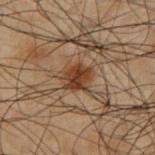Q: Was this lesion biopsied?
A: imaged on a skin check; not biopsied
Q: What are the patient's age and sex?
A: male, aged 48 to 52
Q: What is the imaging modality?
A: total-body-photography crop, ~15 mm field of view
Q: Lesion size?
A: ≈3 mm
Q: What lighting was used for the tile?
A: cross-polarized
Q: What is the anatomic site?
A: the left upper arm
Q: Automated lesion metrics?
A: a nevus-likeness score of about 90/100